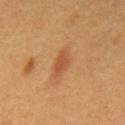{"biopsy_status": "not biopsied; imaged during a skin examination", "site": "mid back", "patient": {"sex": "male", "age_approx": 60}, "lighting": "cross-polarized", "image": {"source": "total-body photography crop", "field_of_view_mm": 15}, "lesion_size": {"long_diameter_mm_approx": 3.0}}